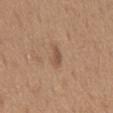{"patient": {"sex": "male", "age_approx": 65}, "lighting": "white-light", "image": {"source": "total-body photography crop", "field_of_view_mm": 15}, "site": "mid back"}